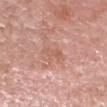biopsy_status: not biopsied; imaged during a skin examination
lighting: white-light
site: head or neck
image:
  source: total-body photography crop
  field_of_view_mm: 15
patient:
  sex: male
  age_approx: 60